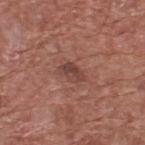Imaged during a routine full-body skin examination; the lesion was not biopsied and no histopathology is available.
A close-up tile cropped from a whole-body skin photograph, about 15 mm across.
The patient is a male aged 73 to 77.
On the upper back.